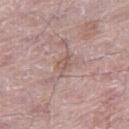Findings:
• subject: male, in their mid- to late 60s
• image: 15 mm crop, total-body photography
• site: the right thigh